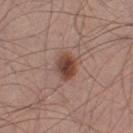Findings:
– biopsy status · total-body-photography surveillance lesion; no biopsy
– lesion size · ≈3.5 mm
– site · the right thigh
– patient · male, aged approximately 55
– imaging modality · ~15 mm crop, total-body skin-cancer survey
– illumination · white-light illumination